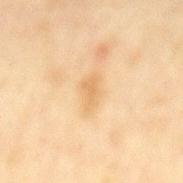Recorded during total-body skin imaging; not selected for excision or biopsy. An algorithmic analysis of the crop reported an area of roughly 5 mm², a shape eccentricity near 0.85, and a symmetry-axis asymmetry near 0.3. Cropped from a total-body skin-imaging series; the visible field is about 15 mm. Captured under cross-polarized illumination. The recorded lesion diameter is about 3.5 mm. Located on the mid back. The patient is a female aged approximately 60.